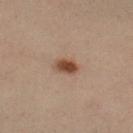Notes:
• biopsy status: no biopsy performed (imaged during a skin exam)
• automated lesion analysis: a within-lesion color-variation index near 3/10 and radial color variation of about 1; a classifier nevus-likeness of about 100/100 and lesion-presence confidence of about 100/100
• acquisition: ~15 mm crop, total-body skin-cancer survey
• patient: female, about 40 years old
• anatomic site: the right lower leg